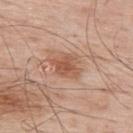  biopsy_status: not biopsied; imaged during a skin examination
  automated_metrics:
    cielab_L: 55
    cielab_a: 23
    cielab_b: 32
    vs_skin_darker_L: 10.0
    vs_skin_contrast_norm: 7.5
    color_variation_0_10: 3.5
    peripheral_color_asymmetry: 1.0
    lesion_detection_confidence_0_100: 100
  image:
    source: total-body photography crop
    field_of_view_mm: 15
  lighting: white-light
  site: back
  lesion_size:
    long_diameter_mm_approx: 3.5
  patient:
    sex: male
    age_approx: 65